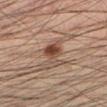Impression: The lesion was photographed on a routine skin check and not biopsied; there is no pathology result. Context: Longest diameter approximately 4 mm. Captured under cross-polarized illumination. Cropped from a total-body skin-imaging series; the visible field is about 15 mm. A male patient, aged around 35. The lesion is on the left lower leg.